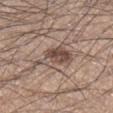Q: Was a biopsy performed?
A: total-body-photography surveillance lesion; no biopsy
Q: What is the lesion's diameter?
A: ≈5.5 mm
Q: How was the tile lit?
A: white-light
Q: Lesion location?
A: the right lower leg
Q: What is the imaging modality?
A: 15 mm crop, total-body photography
Q: What are the patient's age and sex?
A: male, approximately 35 years of age